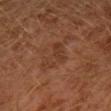Impression: This lesion was catalogued during total-body skin photography and was not selected for biopsy. Clinical summary: Captured under cross-polarized illumination. A region of skin cropped from a whole-body photographic capture, roughly 15 mm wide. The lesion is located on the left leg. The subject is a male aged around 30. The lesion-visualizer software estimated an area of roughly 6.5 mm², an eccentricity of roughly 0.85, and a shape-asymmetry score of about 0.55 (0 = symmetric). It also reported a nevus-likeness score of about 0/100 and a lesion-detection confidence of about 100/100. Longest diameter approximately 4 mm.